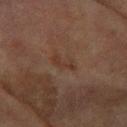{
  "biopsy_status": "not biopsied; imaged during a skin examination",
  "patient": {
    "sex": "female",
    "age_approx": 80
  },
  "lighting": "cross-polarized",
  "lesion_size": {
    "long_diameter_mm_approx": 3.0
  },
  "site": "arm",
  "image": {
    "source": "total-body photography crop",
    "field_of_view_mm": 15
  }
}